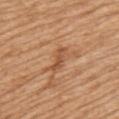Q: Patient demographics?
A: male, aged 68 to 72
Q: How was this image acquired?
A: total-body-photography crop, ~15 mm field of view
Q: What is the anatomic site?
A: the back